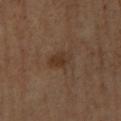Clinical impression: Imaged during a routine full-body skin examination; the lesion was not biopsied and no histopathology is available. Clinical summary: Captured under cross-polarized illumination. The lesion is on the right lower leg. A male subject aged around 65. This image is a 15 mm lesion crop taken from a total-body photograph.